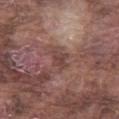Part of a total-body skin-imaging series; this lesion was reviewed on a skin check and was not flagged for biopsy. Automated image analysis of the tile measured an area of roughly 4.5 mm², an eccentricity of roughly 0.75, and a symmetry-axis asymmetry near 0.3. The analysis additionally found an average lesion color of about L≈43 a*≈20 b*≈22 (CIELAB), about 8 CIELAB-L* units darker than the surrounding skin, and a normalized border contrast of about 6.5. The lesion is located on the left thigh. A male patient, aged approximately 75. Cropped from a total-body skin-imaging series; the visible field is about 15 mm. Longest diameter approximately 3 mm. Imaged with white-light lighting.